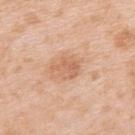| feature | finding |
|---|---|
| follow-up | no biopsy performed (imaged during a skin exam) |
| subject | female, in their 40s |
| diameter | ≈3.5 mm |
| TBP lesion metrics | a footprint of about 5.5 mm², a shape eccentricity near 0.6, and a shape-asymmetry score of about 0.4 (0 = symmetric); a lesion color around L≈63 a*≈22 b*≈34 in CIELAB; a classifier nevus-likeness of about 5/100 |
| acquisition | 15 mm crop, total-body photography |
| body site | the upper back |